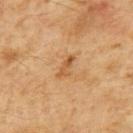Impression:
The lesion was photographed on a routine skin check and not biopsied; there is no pathology result.
Acquisition and patient details:
Cropped from a total-body skin-imaging series; the visible field is about 15 mm. The lesion-visualizer software estimated about 9 CIELAB-L* units darker than the surrounding skin and a normalized lesion–skin contrast near 7. It also reported a nevus-likeness score of about 0/100. A male patient, aged around 60. The lesion is on the mid back.